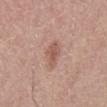Part of a total-body skin-imaging series; this lesion was reviewed on a skin check and was not flagged for biopsy.